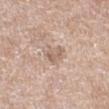| key | value |
|---|---|
| follow-up | catalogued during a skin exam; not biopsied |
| acquisition | ~15 mm crop, total-body skin-cancer survey |
| subject | female, aged 58 to 62 |
| site | the left thigh |
| lighting | white-light |
| automated lesion analysis | a lesion area of about 5 mm², an outline eccentricity of about 0.8 (0 = round, 1 = elongated), and two-axis asymmetry of about 0.3; a border-irregularity index near 3/10 and a peripheral color-asymmetry measure near 1 |
| lesion size | ≈3 mm |